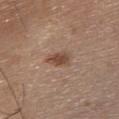Captured during whole-body skin photography for melanoma surveillance; the lesion was not biopsied.
A 15 mm close-up extracted from a 3D total-body photography capture.
This is a white-light tile.
The lesion is located on the chest.
The recorded lesion diameter is about 3 mm.
A female subject aged 63–67.